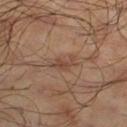Impression: This lesion was catalogued during total-body skin photography and was not selected for biopsy. Context: A male subject aged 63–67. Located on the right lower leg. A close-up tile cropped from a whole-body skin photograph, about 15 mm across.